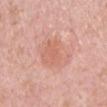This lesion was catalogued during total-body skin photography and was not selected for biopsy. A male subject in their 50s. The lesion is located on the back. The lesion-visualizer software estimated a footprint of about 16 mm², an eccentricity of roughly 0.85, and a shape-asymmetry score of about 0.2 (0 = symmetric). The software also gave a normalized border contrast of about 5. It also reported border irregularity of about 2.5 on a 0–10 scale. The analysis additionally found a classifier nevus-likeness of about 0/100. Imaged with white-light lighting. A roughly 15 mm field-of-view crop from a total-body skin photograph.